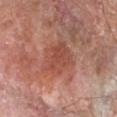Recorded during total-body skin imaging; not selected for excision or biopsy.